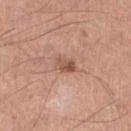Image and clinical context:
Cropped from a whole-body photographic skin survey; the tile spans about 15 mm. The lesion is on the left lower leg. Captured under white-light illumination. A male subject approximately 55 years of age. The lesion's longest dimension is about 2.5 mm.